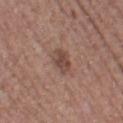Q: Is there a histopathology result?
A: catalogued during a skin exam; not biopsied
Q: Automated lesion metrics?
A: a lesion area of about 5 mm² and an outline eccentricity of about 0.65 (0 = round, 1 = elongated); a border-irregularity rating of about 2/10, internal color variation of about 2.5 on a 0–10 scale, and peripheral color asymmetry of about 1; an automated nevus-likeness rating near 30 out of 100
Q: What kind of image is this?
A: total-body-photography crop, ~15 mm field of view
Q: Lesion size?
A: ≈3 mm
Q: Lesion location?
A: the left thigh
Q: Patient demographics?
A: female, aged 38–42
Q: What lighting was used for the tile?
A: white-light illumination